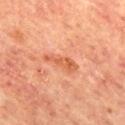notes = imaged on a skin check; not biopsied
anatomic site = the mid back
subject = male, aged approximately 65
acquisition = ~15 mm crop, total-body skin-cancer survey